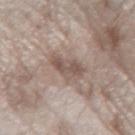Impression: The lesion was photographed on a routine skin check and not biopsied; there is no pathology result. Background: A roughly 15 mm field-of-view crop from a total-body skin photograph. A female subject aged around 75. The lesion is on the left forearm. An algorithmic analysis of the crop reported an outline eccentricity of about 0.7 (0 = round, 1 = elongated) and a symmetry-axis asymmetry near 0.35. And it measured an average lesion color of about L≈53 a*≈14 b*≈22 (CIELAB), about 10 CIELAB-L* units darker than the surrounding skin, and a normalized border contrast of about 7. It also reported a border-irregularity rating of about 3.5/10, internal color variation of about 3.5 on a 0–10 scale, and radial color variation of about 1. The analysis additionally found a nevus-likeness score of about 25/100 and a detector confidence of about 100 out of 100 that the crop contains a lesion. Measured at roughly 3.5 mm in maximum diameter. The tile uses white-light illumination.